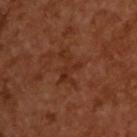Captured during whole-body skin photography for melanoma surveillance; the lesion was not biopsied. A male subject in their mid- to late 60s. The tile uses cross-polarized illumination. A lesion tile, about 15 mm wide, cut from a 3D total-body photograph. Longest diameter approximately 4 mm.